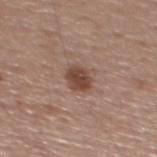No biopsy was performed on this lesion — it was imaged during a full skin examination and was not determined to be concerning. A male patient aged approximately 55. The lesion is on the mid back. Cropped from a whole-body photographic skin survey; the tile spans about 15 mm. Imaged with white-light lighting.